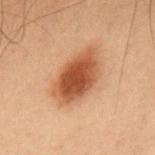{
  "site": "back",
  "image": {
    "source": "total-body photography crop",
    "field_of_view_mm": 15
  },
  "patient": {
    "sex": "male",
    "age_approx": 55
  },
  "lighting": "cross-polarized",
  "lesion_size": {
    "long_diameter_mm_approx": 6.5
  },
  "automated_metrics": {
    "area_mm2_approx": 18.0,
    "shape_asymmetry": 0.2,
    "nevus_likeness_0_100": 100,
    "lesion_detection_confidence_0_100": 100
  }
}